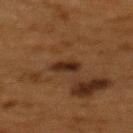Q: Was a biopsy performed?
A: catalogued during a skin exam; not biopsied
Q: What is the imaging modality?
A: ~15 mm crop, total-body skin-cancer survey
Q: What did automated image analysis measure?
A: border irregularity of about 3 on a 0–10 scale, internal color variation of about 3 on a 0–10 scale, and a peripheral color-asymmetry measure near 1; a classifier nevus-likeness of about 75/100
Q: What is the anatomic site?
A: the upper back
Q: What is the lesion's diameter?
A: ~3 mm (longest diameter)
Q: What lighting was used for the tile?
A: cross-polarized
Q: What are the patient's age and sex?
A: female, aged approximately 50